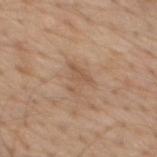Assessment: Captured during whole-body skin photography for melanoma surveillance; the lesion was not biopsied. Acquisition and patient details: Cropped from a whole-body photographic skin survey; the tile spans about 15 mm. The patient is a male approximately 70 years of age. From the chest.